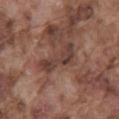* notes — no biopsy performed (imaged during a skin exam)
* image — ~15 mm tile from a whole-body skin photo
* lighting — white-light illumination
* TBP lesion metrics — an area of roughly 6.5 mm², a shape eccentricity near 0.95, and a shape-asymmetry score of about 0.55 (0 = symmetric); a color-variation rating of about 3/10
* anatomic site — the mid back
* lesion diameter — ≈5 mm
* patient — male, in their mid-70s